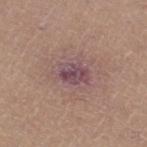follow-up: no biopsy performed (imaged during a skin exam) | lesion diameter: about 5 mm | subject: female, aged approximately 45 | body site: the leg | lighting: white-light illumination | imaging modality: ~15 mm tile from a whole-body skin photo.